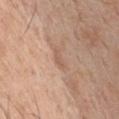Q: Illumination type?
A: white-light
Q: What is the lesion's diameter?
A: ~2.5 mm (longest diameter)
Q: Automated lesion metrics?
A: an average lesion color of about L≈58 a*≈19 b*≈29 (CIELAB), about 7 CIELAB-L* units darker than the surrounding skin, and a normalized lesion–skin contrast near 4.5; a border-irregularity index near 3.5/10, a color-variation rating of about 0/10, and a peripheral color-asymmetry measure near 0; lesion-presence confidence of about 70/100
Q: Patient demographics?
A: male, aged around 60
Q: What is the imaging modality?
A: 15 mm crop, total-body photography
Q: Where on the body is the lesion?
A: the chest